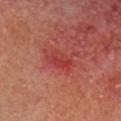notes = total-body-photography surveillance lesion; no biopsy | subject = male, aged 63 to 67 | image = total-body-photography crop, ~15 mm field of view | TBP lesion metrics = an area of roughly 5.5 mm² and two-axis asymmetry of about 0.25; internal color variation of about 4 on a 0–10 scale and radial color variation of about 1.5 | location = the head or neck | lesion size = ~3 mm (longest diameter) | tile lighting = cross-polarized illumination.